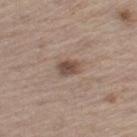follow-up=total-body-photography surveillance lesion; no biopsy